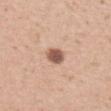- notes · imaged on a skin check; not biopsied
- location · the upper back
- image · 15 mm crop, total-body photography
- diameter · about 3 mm
- patient · female, aged around 40